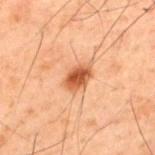Impression: Recorded during total-body skin imaging; not selected for excision or biopsy. Acquisition and patient details: Automated tile analysis of the lesion measured a footprint of about 6 mm². The analysis additionally found an average lesion color of about L≈44 a*≈23 b*≈33 (CIELAB), about 13 CIELAB-L* units darker than the surrounding skin, and a lesion-to-skin contrast of about 10 (normalized; higher = more distinct). It also reported border irregularity of about 2 on a 0–10 scale, a within-lesion color-variation index near 4/10, and a peripheral color-asymmetry measure near 1.5. And it measured an automated nevus-likeness rating near 100 out of 100 and a detector confidence of about 100 out of 100 that the crop contains a lesion. A male patient about 60 years old. On the upper back. A close-up tile cropped from a whole-body skin photograph, about 15 mm across. Measured at roughly 3.5 mm in maximum diameter.